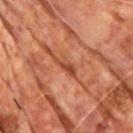Notes:
- workup — catalogued during a skin exam; not biopsied
- subject — male, aged 58–62
- lesion diameter — ~3.5 mm (longest diameter)
- image source — ~15 mm tile from a whole-body skin photo
- anatomic site — the back
- image-analysis metrics — a footprint of about 3.5 mm², a shape eccentricity near 0.95, and a shape-asymmetry score of about 0.35 (0 = symmetric); a border-irregularity index near 4.5/10, a color-variation rating of about 2/10, and peripheral color asymmetry of about 0.5; a detector confidence of about 65 out of 100 that the crop contains a lesion
- illumination — cross-polarized illumination